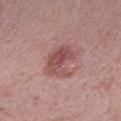Q: Was a biopsy performed?
A: catalogued during a skin exam; not biopsied
Q: Where on the body is the lesion?
A: the arm
Q: What is the lesion's diameter?
A: ≈4 mm
Q: What are the patient's age and sex?
A: male, approximately 40 years of age
Q: How was this image acquired?
A: total-body-photography crop, ~15 mm field of view
Q: What lighting was used for the tile?
A: white-light